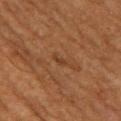notes: catalogued during a skin exam; not biopsied
illumination: cross-polarized
patient: male, in their 60s
acquisition: ~15 mm tile from a whole-body skin photo
TBP lesion metrics: a mean CIELAB color near L≈36 a*≈20 b*≈31, roughly 6 lightness units darker than nearby skin, and a lesion-to-skin contrast of about 5 (normalized; higher = more distinct); border irregularity of about 4 on a 0–10 scale, a color-variation rating of about 0/10, and a peripheral color-asymmetry measure near 0
body site: the back